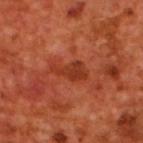This lesion was catalogued during total-body skin photography and was not selected for biopsy. The patient is a male about 70 years old. A 15 mm close-up extracted from a 3D total-body photography capture. The lesion is on the upper back.